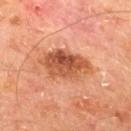Impression:
The lesion was photographed on a routine skin check and not biopsied; there is no pathology result.
Background:
Captured under cross-polarized illumination. On the mid back. A 15 mm crop from a total-body photograph taken for skin-cancer surveillance. Approximately 6.5 mm at its widest. A male subject approximately 65 years of age. Automated image analysis of the tile measured a color-variation rating of about 7/10. The software also gave an automated nevus-likeness rating near 45 out of 100.